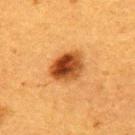No biopsy was performed on this lesion — it was imaged during a full skin examination and was not determined to be concerning. Imaged with cross-polarized lighting. The total-body-photography lesion software estimated an eccentricity of roughly 0.6 and a shape-asymmetry score of about 0.2 (0 = symmetric). And it measured an automated nevus-likeness rating near 100 out of 100 and lesion-presence confidence of about 100/100. A roughly 15 mm field-of-view crop from a total-body skin photograph. The lesion is located on the upper back. A female patient, in their 40s.